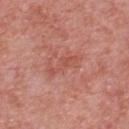Notes:
– notes — total-body-photography surveillance lesion; no biopsy
– size — ≈4 mm
– imaging modality — 15 mm crop, total-body photography
– site — the chest
– subject — male, roughly 70 years of age